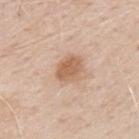{
  "biopsy_status": "not biopsied; imaged during a skin examination",
  "image": {
    "source": "total-body photography crop",
    "field_of_view_mm": 15
  },
  "patient": {
    "sex": "male",
    "age_approx": 70
  },
  "lighting": "white-light",
  "site": "chest",
  "automated_metrics": {
    "cielab_L": 61,
    "cielab_a": 19,
    "cielab_b": 33,
    "vs_skin_darker_L": 11.0,
    "nevus_likeness_0_100": 15
  }
}